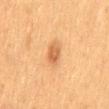Q: Is there a histopathology result?
A: catalogued during a skin exam; not biopsied
Q: Automated lesion metrics?
A: roughly 9 lightness units darker than nearby skin and a normalized lesion–skin contrast near 6.5; a within-lesion color-variation index near 3.5/10 and radial color variation of about 1
Q: How was this image acquired?
A: total-body-photography crop, ~15 mm field of view
Q: What lighting was used for the tile?
A: cross-polarized
Q: Lesion location?
A: the back
Q: What is the lesion's diameter?
A: ≈3.5 mm
Q: Who is the patient?
A: male, aged 48–52